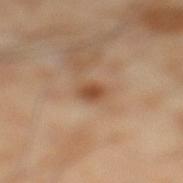Impression: The lesion was photographed on a routine skin check and not biopsied; there is no pathology result. Clinical summary: A male patient, about 55 years old. Cropped from a whole-body photographic skin survey; the tile spans about 15 mm. From the left lower leg.